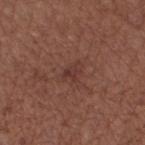  biopsy_status: not biopsied; imaged during a skin examination
  image:
    source: total-body photography crop
    field_of_view_mm: 15
  patient:
    sex: female
    age_approx: 55
  site: right thigh